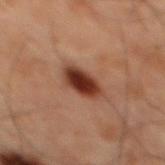| field | value |
|---|---|
| biopsy status | imaged on a skin check; not biopsied |
| size | ≈3.5 mm |
| lighting | cross-polarized |
| image source | ~15 mm crop, total-body skin-cancer survey |
| subject | male, aged approximately 60 |
| site | the mid back |